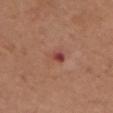follow-up = no biopsy performed (imaged during a skin exam) | lesion size = ~3.5 mm (longest diameter) | body site = the chest | TBP lesion metrics = a lesion area of about 6.5 mm²; border irregularity of about 3 on a 0–10 scale and a color-variation rating of about 9.5/10 | image = ~15 mm crop, total-body skin-cancer survey | patient = female, aged 63 to 67.